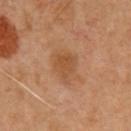Assessment: Captured during whole-body skin photography for melanoma surveillance; the lesion was not biopsied. Acquisition and patient details: A lesion tile, about 15 mm wide, cut from a 3D total-body photograph. The patient is a male aged around 50. The tile uses cross-polarized illumination. The lesion is located on the chest. Approximately 3.5 mm at its widest. Automated image analysis of the tile measured an area of roughly 6.5 mm², an eccentricity of roughly 0.6, and a symmetry-axis asymmetry near 0.35.